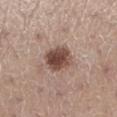This lesion was catalogued during total-body skin photography and was not selected for biopsy. A female subject approximately 45 years of age. The lesion is located on the left lower leg. Captured under white-light illumination. Measured at roughly 4 mm in maximum diameter. A roughly 15 mm field-of-view crop from a total-body skin photograph.